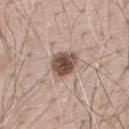The lesion was tiled from a total-body skin photograph and was not biopsied. A male subject roughly 70 years of age. On the upper back. A 15 mm close-up extracted from a 3D total-body photography capture. The total-body-photography lesion software estimated an average lesion color of about L≈50 a*≈17 b*≈24 (CIELAB), roughly 17 lightness units darker than nearby skin, and a normalized border contrast of about 11. And it measured a nevus-likeness score of about 95/100 and lesion-presence confidence of about 100/100.